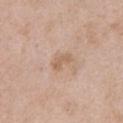{
  "biopsy_status": "not biopsied; imaged during a skin examination",
  "patient": {
    "sex": "male",
    "age_approx": 50
  },
  "image": {
    "source": "total-body photography crop",
    "field_of_view_mm": 15
  },
  "lighting": "white-light",
  "site": "chest",
  "automated_metrics": {
    "area_mm2_approx": 3.5,
    "eccentricity": 0.85,
    "shape_asymmetry": 0.35,
    "cielab_L": 61,
    "cielab_a": 17,
    "cielab_b": 31,
    "vs_skin_darker_L": 8.0,
    "vs_skin_contrast_norm": 6.0,
    "nevus_likeness_0_100": 0,
    "lesion_detection_confidence_0_100": 100
  },
  "lesion_size": {
    "long_diameter_mm_approx": 2.5
  }
}